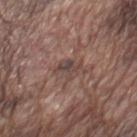Imaged with white-light lighting.
Cropped from a total-body skin-imaging series; the visible field is about 15 mm.
Measured at roughly 3 mm in maximum diameter.
The lesion is on the chest.
The subject is a male aged 73 to 77.